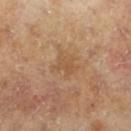<case>
  <biopsy_status>not biopsied; imaged during a skin examination</biopsy_status>
  <site>right lower leg</site>
  <lesion_size>
    <long_diameter_mm_approx>3.0</long_diameter_mm_approx>
  </lesion_size>
  <lighting>cross-polarized</lighting>
  <automated_metrics>
    <cielab_L>54</cielab_L>
    <cielab_a>18</cielab_a>
    <cielab_b>35</cielab_b>
    <vs_skin_darker_L>6.0</vs_skin_darker_L>
    <vs_skin_contrast_norm>5.0</vs_skin_contrast_norm>
    <nevus_likeness_0_100>0</nevus_likeness_0_100>
  </automated_metrics>
  <image>
    <source>total-body photography crop</source>
    <field_of_view_mm>15</field_of_view_mm>
  </image>
  <patient>
    <sex>male</sex>
    <age_approx>65</age_approx>
  </patient>
</case>